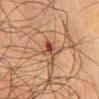<tbp_lesion>
  <biopsy_status>not biopsied; imaged during a skin examination</biopsy_status>
  <site>chest</site>
  <lesion_size>
    <long_diameter_mm_approx>2.5</long_diameter_mm_approx>
  </lesion_size>
  <patient>
    <sex>male</sex>
    <age_approx>70</age_approx>
  </patient>
  <lighting>cross-polarized</lighting>
  <image>
    <source>total-body photography crop</source>
    <field_of_view_mm>15</field_of_view_mm>
  </image>
</tbp_lesion>